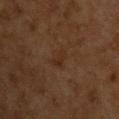| feature | finding |
|---|---|
| image source | 15 mm crop, total-body photography |
| tile lighting | cross-polarized |
| diameter | ~3 mm (longest diameter) |
| body site | the back |
| subject | male, roughly 60 years of age |